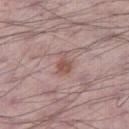biopsy status: total-body-photography surveillance lesion; no biopsy | image: ~15 mm crop, total-body skin-cancer survey | subject: male, approximately 70 years of age | site: the right thigh.